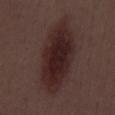The lesion was tiled from a total-body skin photograph and was not biopsied.
A male patient, in their mid-50s.
About 10 mm across.
A 15 mm close-up tile from a total-body photography series done for melanoma screening.
The lesion is on the mid back.
The lesion-visualizer software estimated an area of roughly 32 mm², a shape eccentricity near 0.9, and two-axis asymmetry of about 0.15. The software also gave a border-irregularity index near 2.5/10, internal color variation of about 4 on a 0–10 scale, and a peripheral color-asymmetry measure near 1.
Captured under white-light illumination.